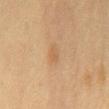Case summary:
- follow-up — imaged on a skin check; not biopsied
- tile lighting — cross-polarized
- automated lesion analysis — two-axis asymmetry of about 0.3; a border-irregularity index near 2.5/10, internal color variation of about 2 on a 0–10 scale, and a peripheral color-asymmetry measure near 1
- subject — female, aged 48–52
- image — ~15 mm crop, total-body skin-cancer survey
- lesion size — about 3 mm
- body site — the chest